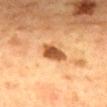Impression:
Imaged during a routine full-body skin examination; the lesion was not biopsied and no histopathology is available.
Background:
On the mid back. A female patient, about 60 years old. The tile uses cross-polarized illumination. About 3 mm across. A 15 mm close-up tile from a total-body photography series done for melanoma screening.